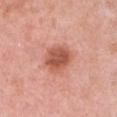<case>
  <lighting>white-light</lighting>
  <image>
    <source>total-body photography crop</source>
    <field_of_view_mm>15</field_of_view_mm>
  </image>
  <patient>
    <sex>female</sex>
    <age_approx>40</age_approx>
  </patient>
  <site>chest</site>
  <automated_metrics>
    <border_irregularity_0_10>1.5</border_irregularity_0_10>
    <color_variation_0_10>4.0</color_variation_0_10>
    <peripheral_color_asymmetry>1.0</peripheral_color_asymmetry>
    <lesion_detection_confidence_0_100>100</lesion_detection_confidence_0_100>
  </automated_metrics>
</case>